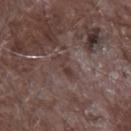Case summary:
• biopsy status: catalogued during a skin exam; not biopsied
• body site: the left forearm
• image: ~15 mm tile from a whole-body skin photo
• subject: male, in their mid- to late 60s